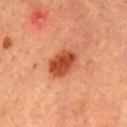Background:
Located on the chest. Imaged with cross-polarized lighting. Cropped from a total-body skin-imaging series; the visible field is about 15 mm. A male patient, aged around 65. The total-body-photography lesion software estimated a lesion area of about 9 mm², an outline eccentricity of about 0.7 (0 = round, 1 = elongated), and a shape-asymmetry score of about 0.15 (0 = symmetric). The software also gave an average lesion color of about L≈45 a*≈31 b*≈36 (CIELAB), roughly 13 lightness units darker than nearby skin, and a normalized lesion–skin contrast near 10. The software also gave a border-irregularity rating of about 1.5/10 and internal color variation of about 4 on a 0–10 scale. The software also gave a classifier nevus-likeness of about 100/100 and lesion-presence confidence of about 100/100. The recorded lesion diameter is about 4 mm.